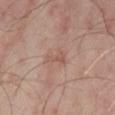Clinical impression:
The lesion was tiled from a total-body skin photograph and was not biopsied.
Image and clinical context:
From the abdomen. The total-body-photography lesion software estimated a normalized lesion–skin contrast near 5. And it measured a border-irregularity index near 5.5/10, internal color variation of about 1 on a 0–10 scale, and radial color variation of about 0. This image is a 15 mm lesion crop taken from a total-body photograph. The tile uses cross-polarized illumination. A male subject in their 50s. The recorded lesion diameter is about 3 mm.